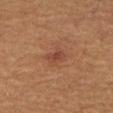Imaged during a routine full-body skin examination; the lesion was not biopsied and no histopathology is available.
The lesion is located on the right thigh.
The total-body-photography lesion software estimated an eccentricity of roughly 0.85 and a symmetry-axis asymmetry near 0.3. It also reported a lesion–skin lightness drop of about 7 and a normalized lesion–skin contrast near 6. And it measured radial color variation of about 0.5. It also reported a classifier nevus-likeness of about 20/100 and a detector confidence of about 100 out of 100 that the crop contains a lesion.
Measured at roughly 2.5 mm in maximum diameter.
A male patient, roughly 60 years of age.
This image is a 15 mm lesion crop taken from a total-body photograph.
This is a cross-polarized tile.